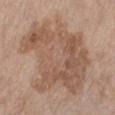Part of a total-body skin-imaging series; this lesion was reviewed on a skin check and was not flagged for biopsy.
The lesion-visualizer software estimated a lesion color around L≈55 a*≈17 b*≈28 in CIELAB, a lesion–skin lightness drop of about 9, and a normalized border contrast of about 6.5. And it measured a border-irregularity index near 5.5/10, internal color variation of about 5 on a 0–10 scale, and radial color variation of about 1.5. The analysis additionally found an automated nevus-likeness rating near 0 out of 100 and a detector confidence of about 100 out of 100 that the crop contains a lesion.
The lesion's longest dimension is about 11 mm.
Cropped from a whole-body photographic skin survey; the tile spans about 15 mm.
This is a white-light tile.
Located on the right lower leg.
A female subject approximately 65 years of age.